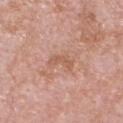Notes:
* biopsy status: no biopsy performed (imaged during a skin exam)
* site: the chest
* patient: male, aged around 55
* image source: ~15 mm tile from a whole-body skin photo
* illumination: white-light illumination